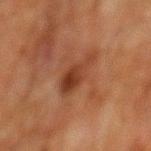patient = male, aged 78 to 82; body site = the mid back; image = ~15 mm tile from a whole-body skin photo.